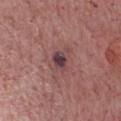Q: Is there a histopathology result?
A: catalogued during a skin exam; not biopsied
Q: What is the imaging modality?
A: 15 mm crop, total-body photography
Q: What did automated image analysis measure?
A: a lesion area of about 4.5 mm² and a symmetry-axis asymmetry near 0.2; border irregularity of about 2 on a 0–10 scale and radial color variation of about 2
Q: Where on the body is the lesion?
A: the chest
Q: What are the patient's age and sex?
A: male, approximately 75 years of age